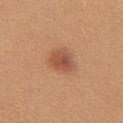Clinical impression:
The lesion was photographed on a routine skin check and not biopsied; there is no pathology result.
Clinical summary:
The lesion's longest dimension is about 3.5 mm. On the front of the torso. A lesion tile, about 15 mm wide, cut from a 3D total-body photograph. The subject is a female in their mid-20s. The total-body-photography lesion software estimated an area of roughly 7.5 mm² and an eccentricity of roughly 0.65.